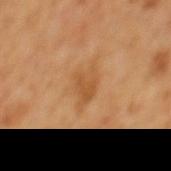A male subject approximately 65 years of age. The lesion-visualizer software estimated an area of roughly 7.5 mm² and a shape-asymmetry score of about 0.3 (0 = symmetric). And it measured a lesion color around L≈52 a*≈22 b*≈39 in CIELAB and a lesion–skin lightness drop of about 7. The analysis additionally found border irregularity of about 3.5 on a 0–10 scale, a color-variation rating of about 3/10, and a peripheral color-asymmetry measure near 1. It also reported a nevus-likeness score of about 0/100. Located on the mid back. The recorded lesion diameter is about 4.5 mm. Captured under cross-polarized illumination. Cropped from a whole-body photographic skin survey; the tile spans about 15 mm.